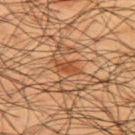No biopsy was performed on this lesion — it was imaged during a full skin examination and was not determined to be concerning. On the back. Captured under cross-polarized illumination. An algorithmic analysis of the crop reported a shape eccentricity near 0.9 and a shape-asymmetry score of about 0.3 (0 = symmetric). The analysis additionally found a lesion-detection confidence of about 90/100. The subject is a male aged 58 to 62. A close-up tile cropped from a whole-body skin photograph, about 15 mm across.